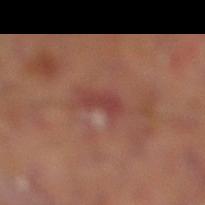Findings:
* biopsy status — no biopsy performed (imaged during a skin exam)
* tile lighting — cross-polarized
* automated metrics — internal color variation of about 1 on a 0–10 scale and a peripheral color-asymmetry measure near 0; a nevus-likeness score of about 0/100 and a lesion-detection confidence of about 90/100
* diameter — about 3.5 mm
* image — total-body-photography crop, ~15 mm field of view
* anatomic site — the right lower leg
* patient — male, roughly 65 years of age